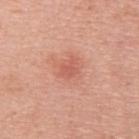Imaged during a routine full-body skin examination; the lesion was not biopsied and no histopathology is available.